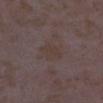follow-up = no biopsy performed (imaged during a skin exam) | anatomic site = the right lower leg | image-analysis metrics = a lesion color around L≈37 a*≈12 b*≈18 in CIELAB and about 4 CIELAB-L* units darker than the surrounding skin; an automated nevus-likeness rating near 0 out of 100 and a lesion-detection confidence of about 100/100 | acquisition = ~15 mm crop, total-body skin-cancer survey | subject = female, approximately 35 years of age.